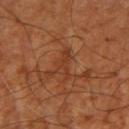Findings:
– workup: no biopsy performed (imaged during a skin exam)
– diameter: ≈3.5 mm
– patient: aged around 65
– tile lighting: cross-polarized illumination
– image source: total-body-photography crop, ~15 mm field of view
– site: the right upper arm
– TBP lesion metrics: a footprint of about 4 mm² and an eccentricity of roughly 0.9; a border-irregularity index near 6/10 and a color-variation rating of about 0/10; a detector confidence of about 85 out of 100 that the crop contains a lesion